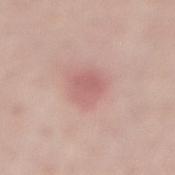- follow-up: total-body-photography surveillance lesion; no biopsy
- illumination: white-light illumination
- subject: male, aged 58–62
- anatomic site: the lower back
- lesion size: ≈3.5 mm
- image: ~15 mm tile from a whole-body skin photo